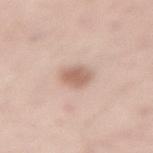{"biopsy_status": "not biopsied; imaged during a skin examination", "lighting": "white-light", "site": "lower back", "lesion_size": {"long_diameter_mm_approx": 2.5}, "patient": {"sex": "male", "age_approx": 55}, "automated_metrics": {"cielab_L": 62, "cielab_a": 18, "cielab_b": 28, "vs_skin_darker_L": 12.0, "border_irregularity_0_10": 1.5, "color_variation_0_10": 2.5, "nevus_likeness_0_100": 85, "lesion_detection_confidence_0_100": 100}, "image": {"source": "total-body photography crop", "field_of_view_mm": 15}}